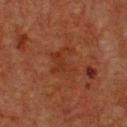biopsy status: no biopsy performed (imaged during a skin exam)
lesion size: ≈4 mm
lighting: cross-polarized illumination
patient: male, aged approximately 75
imaging modality: ~15 mm crop, total-body skin-cancer survey
location: the chest